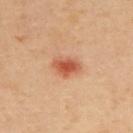Acquisition and patient details:
Automated tile analysis of the lesion measured a mean CIELAB color near L≈57 a*≈29 b*≈36, about 13 CIELAB-L* units darker than the surrounding skin, and a normalized lesion–skin contrast near 8.5. The analysis additionally found border irregularity of about 2.5 on a 0–10 scale, internal color variation of about 4 on a 0–10 scale, and radial color variation of about 1. Captured under cross-polarized illumination. A female subject, aged 38–42. From the back. A 15 mm close-up tile from a total-body photography series done for melanoma screening. About 3.5 mm across.